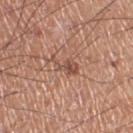Q: Was this lesion biopsied?
A: catalogued during a skin exam; not biopsied
Q: What kind of image is this?
A: total-body-photography crop, ~15 mm field of view
Q: What is the anatomic site?
A: the right thigh
Q: How large is the lesion?
A: about 3.5 mm
Q: Illumination type?
A: white-light
Q: Patient demographics?
A: male, approximately 55 years of age